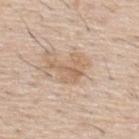Case summary:
– notes — imaged on a skin check; not biopsied
– subject — male, in their mid- to late 50s
– body site — the upper back
– lesion size — ~4 mm (longest diameter)
– illumination — white-light illumination
– image source — 15 mm crop, total-body photography
– automated lesion analysis — a border-irregularity rating of about 9.5/10, a color-variation rating of about 2.5/10, and a peripheral color-asymmetry measure near 1; a detector confidence of about 100 out of 100 that the crop contains a lesion